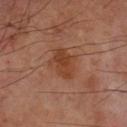<tbp_lesion>
<biopsy_status>not biopsied; imaged during a skin examination</biopsy_status>
<image>
  <source>total-body photography crop</source>
  <field_of_view_mm>15</field_of_view_mm>
</image>
<site>arm</site>
<patient>
  <sex>male</sex>
  <age_approx>70</age_approx>
</patient>
<lesion_size>
  <long_diameter_mm_approx>4.0</long_diameter_mm_approx>
</lesion_size>
<automated_metrics>
  <border_irregularity_0_10>3.0</border_irregularity_0_10>
  <color_variation_0_10>3.0</color_variation_0_10>
  <peripheral_color_asymmetry>1.0</peripheral_color_asymmetry>
  <nevus_likeness_0_100>45</nevus_likeness_0_100>
  <lesion_detection_confidence_0_100>100</lesion_detection_confidence_0_100>
</automated_metrics>
</tbp_lesion>